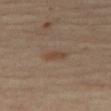Captured during whole-body skin photography for melanoma surveillance; the lesion was not biopsied.
The total-body-photography lesion software estimated a within-lesion color-variation index near 1/10 and a peripheral color-asymmetry measure near 0.5.
A female patient, approximately 65 years of age.
The lesion is on the right thigh.
A 15 mm close-up extracted from a 3D total-body photography capture.